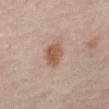Clinical impression:
This lesion was catalogued during total-body skin photography and was not selected for biopsy.
Context:
A lesion tile, about 15 mm wide, cut from a 3D total-body photograph. A patient aged 53–57. On the abdomen. Automated tile analysis of the lesion measured a color-variation rating of about 4/10 and radial color variation of about 1. The recorded lesion diameter is about 3.5 mm.